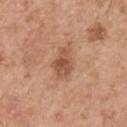The lesion was tiled from a total-body skin photograph and was not biopsied. A roughly 15 mm field-of-view crop from a total-body skin photograph. The tile uses white-light illumination. The lesion is located on the right upper arm. A male subject, roughly 55 years of age. Automated image analysis of the tile measured a lesion area of about 7 mm², an eccentricity of roughly 0.75, and a symmetry-axis asymmetry near 0.25. And it measured an average lesion color of about L≈53 a*≈23 b*≈33 (CIELAB). The software also gave lesion-presence confidence of about 100/100.